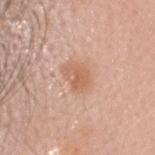No biopsy was performed on this lesion — it was imaged during a full skin examination and was not determined to be concerning. On the head or neck. Automated tile analysis of the lesion measured a footprint of about 5.5 mm², an eccentricity of roughly 0.7, and a symmetry-axis asymmetry near 0.3. The software also gave about 8 CIELAB-L* units darker than the surrounding skin and a normalized lesion–skin contrast near 6.5. A region of skin cropped from a whole-body photographic capture, roughly 15 mm wide. A male subject, aged approximately 35.